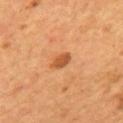| feature | finding |
|---|---|
| notes | catalogued during a skin exam; not biopsied |
| image-analysis metrics | a footprint of about 3.5 mm² and a shape-asymmetry score of about 0.25 (0 = symmetric); a mean CIELAB color near L≈50 a*≈25 b*≈39 and a normalized lesion–skin contrast near 7.5; a border-irregularity rating of about 2/10, a color-variation rating of about 1.5/10, and peripheral color asymmetry of about 0.5; a nevus-likeness score of about 80/100 |
| lesion size | ≈2.5 mm |
| acquisition | ~15 mm crop, total-body skin-cancer survey |
| subject | male, in their mid- to late 50s |
| body site | the back |
| tile lighting | cross-polarized illumination |